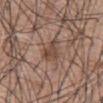Assessment: The lesion was tiled from a total-body skin photograph and was not biopsied. Context: Located on the abdomen. A 15 mm crop from a total-body photograph taken for skin-cancer surveillance. The subject is a male aged 58 to 62. Automated tile analysis of the lesion measured a footprint of about 3.5 mm², an eccentricity of roughly 0.65, and two-axis asymmetry of about 0.5. And it measured border irregularity of about 5.5 on a 0–10 scale, internal color variation of about 2.5 on a 0–10 scale, and radial color variation of about 1. The software also gave an automated nevus-likeness rating near 5 out of 100.